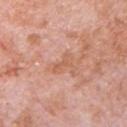Imaged during a routine full-body skin examination; the lesion was not biopsied and no histopathology is available. The lesion is on the chest. A male subject, roughly 80 years of age. The recorded lesion diameter is about 3 mm. A region of skin cropped from a whole-body photographic capture, roughly 15 mm wide. Imaged with white-light lighting. Automated tile analysis of the lesion measured an eccentricity of roughly 0.85 and a shape-asymmetry score of about 0.35 (0 = symmetric). And it measured a mean CIELAB color near L≈60 a*≈24 b*≈33, about 6 CIELAB-L* units darker than the surrounding skin, and a normalized lesion–skin contrast near 5. And it measured lesion-presence confidence of about 100/100.